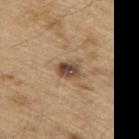This lesion was catalogued during total-body skin photography and was not selected for biopsy. From the upper back. A male patient aged 68 to 72. This is a white-light tile. An algorithmic analysis of the crop reported a border-irregularity rating of about 2.5/10, internal color variation of about 6.5 on a 0–10 scale, and radial color variation of about 2.5. The analysis additionally found a detector confidence of about 100 out of 100 that the crop contains a lesion. A roughly 15 mm field-of-view crop from a total-body skin photograph. Measured at roughly 3 mm in maximum diameter.